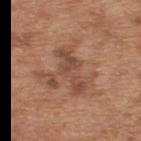notes=imaged on a skin check; not biopsied
image source=15 mm crop, total-body photography
location=the upper back
patient=male, approximately 65 years of age